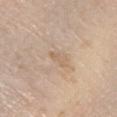biopsy_status: not biopsied; imaged during a skin examination
site: right lower leg
image:
  source: total-body photography crop
  field_of_view_mm: 15
lesion_size:
  long_diameter_mm_approx: 3.0
patient:
  sex: female
  age_approx: 70
automated_metrics:
  border_irregularity_0_10: 4.0
  color_variation_0_10: 0.0
  peripheral_color_asymmetry: 0.0
lighting: white-light